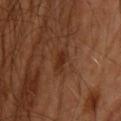Imaged during a routine full-body skin examination; the lesion was not biopsied and no histopathology is available.
The total-body-photography lesion software estimated a border-irregularity rating of about 3/10, a within-lesion color-variation index near 1.5/10, and radial color variation of about 0.5. The software also gave a nevus-likeness score of about 10/100 and a detector confidence of about 100 out of 100 that the crop contains a lesion.
This is a cross-polarized tile.
A 15 mm crop from a total-body photograph taken for skin-cancer surveillance.
Located on the head or neck.
A male subject, approximately 40 years of age.